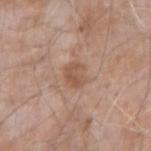<record>
<biopsy_status>not biopsied; imaged during a skin examination</biopsy_status>
<image>
  <source>total-body photography crop</source>
  <field_of_view_mm>15</field_of_view_mm>
</image>
<site>right forearm</site>
<lesion_size>
  <long_diameter_mm_approx>3.0</long_diameter_mm_approx>
</lesion_size>
<patient>
  <sex>male</sex>
  <age_approx>75</age_approx>
</patient>
<lighting>white-light</lighting>
</record>